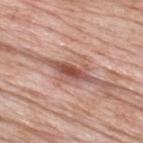<tbp_lesion>
<image>
  <source>total-body photography crop</source>
  <field_of_view_mm>15</field_of_view_mm>
</image>
<patient>
  <sex>male</sex>
  <age_approx>60</age_approx>
</patient>
<site>upper back</site>
</tbp_lesion>